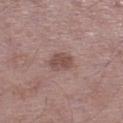Q: Was a biopsy performed?
A: imaged on a skin check; not biopsied
Q: What are the patient's age and sex?
A: male, aged approximately 50
Q: Lesion location?
A: the left lower leg
Q: How was this image acquired?
A: 15 mm crop, total-body photography